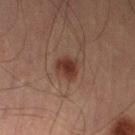Impression:
The lesion was tiled from a total-body skin photograph and was not biopsied.
Clinical summary:
The lesion's longest dimension is about 3 mm. A male patient aged 53–57. Automated tile analysis of the lesion measured an area of roughly 6 mm², an eccentricity of roughly 0.5, and a symmetry-axis asymmetry near 0.15. It also reported a mean CIELAB color near L≈30 a*≈19 b*≈22, a lesion–skin lightness drop of about 9, and a lesion-to-skin contrast of about 9 (normalized; higher = more distinct). And it measured border irregularity of about 1.5 on a 0–10 scale, a color-variation rating of about 2.5/10, and a peripheral color-asymmetry measure near 0.5. Imaged with cross-polarized lighting. This image is a 15 mm lesion crop taken from a total-body photograph. The lesion is located on the leg.